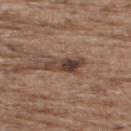Impression: This lesion was catalogued during total-body skin photography and was not selected for biopsy. Acquisition and patient details: An algorithmic analysis of the crop reported a lesion color around L≈40 a*≈17 b*≈24 in CIELAB, roughly 13 lightness units darker than nearby skin, and a normalized lesion–skin contrast near 10.5. And it measured a within-lesion color-variation index near 4.5/10. Measured at roughly 4.5 mm in maximum diameter. A male patient aged 68 to 72. A 15 mm crop from a total-body photograph taken for skin-cancer surveillance. Located on the upper back.